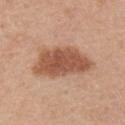Part of a total-body skin-imaging series; this lesion was reviewed on a skin check and was not flagged for biopsy.
Longest diameter approximately 7.5 mm.
A male subject, in their 30s.
This is a white-light tile.
A region of skin cropped from a whole-body photographic capture, roughly 15 mm wide.
The lesion is on the right upper arm.
Automated tile analysis of the lesion measured an area of roughly 21 mm², an eccentricity of roughly 0.85, and two-axis asymmetry of about 0.3. The analysis additionally found an average lesion color of about L≈53 a*≈23 b*≈32 (CIELAB) and about 14 CIELAB-L* units darker than the surrounding skin.